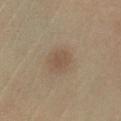Clinical impression: Part of a total-body skin-imaging series; this lesion was reviewed on a skin check and was not flagged for biopsy. Background: Approximately 3 mm at its widest. Located on the left lower leg. The subject is a male aged approximately 60. A 15 mm close-up tile from a total-body photography series done for melanoma screening.